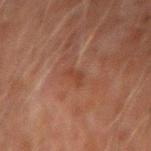Clinical impression:
No biopsy was performed on this lesion — it was imaged during a full skin examination and was not determined to be concerning.
Context:
Captured under cross-polarized illumination. A roughly 15 mm field-of-view crop from a total-body skin photograph. A male subject about 60 years old. The lesion is on the left forearm.